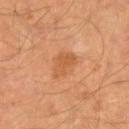The lesion was tiled from a total-body skin photograph and was not biopsied.
The subject is a male about 40 years old.
A 15 mm crop from a total-body photograph taken for skin-cancer surveillance.
The lesion is located on the right lower leg.